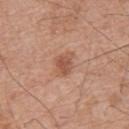Impression:
This lesion was catalogued during total-body skin photography and was not selected for biopsy.
Background:
Imaged with white-light lighting. From the upper back. A roughly 15 mm field-of-view crop from a total-body skin photograph. An algorithmic analysis of the crop reported a border-irregularity rating of about 3.5/10 and a color-variation rating of about 2.5/10. The software also gave an automated nevus-likeness rating near 45 out of 100 and a detector confidence of about 100 out of 100 that the crop contains a lesion. A male patient, approximately 65 years of age. Approximately 3 mm at its widest.